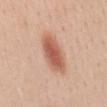A female patient roughly 45 years of age. The tile uses white-light illumination. The lesion's longest dimension is about 6 mm. The total-body-photography lesion software estimated an area of roughly 12 mm², an eccentricity of roughly 0.9, and a symmetry-axis asymmetry near 0.15. It also reported a border-irregularity index near 2/10, a within-lesion color-variation index near 4/10, and a peripheral color-asymmetry measure near 1. Located on the mid back. Cropped from a whole-body photographic skin survey; the tile spans about 15 mm.